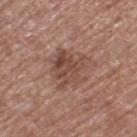| feature | finding |
|---|---|
| notes | imaged on a skin check; not biopsied |
| size | ~5 mm (longest diameter) |
| body site | the leg |
| image | 15 mm crop, total-body photography |
| subject | female, in their mid- to late 70s |
| illumination | white-light illumination |